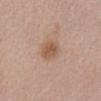A 15 mm close-up tile from a total-body photography series done for melanoma screening. The patient is a female aged approximately 70. The lesion is on the abdomen. This is a white-light tile. The total-body-photography lesion software estimated an area of roughly 5.5 mm², a shape eccentricity near 0.75, and two-axis asymmetry of about 0.2. The software also gave a lesion color around L≈55 a*≈19 b*≈31 in CIELAB and a normalized border contrast of about 7.5. It also reported border irregularity of about 2 on a 0–10 scale, internal color variation of about 2.5 on a 0–10 scale, and a peripheral color-asymmetry measure near 1.